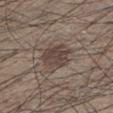Notes:
– biopsy status: imaged on a skin check; not biopsied
– subject: male, aged approximately 35
– site: the left lower leg
– lesion size: ≈4.5 mm
– image-analysis metrics: an area of roughly 12 mm², a shape eccentricity near 0.7, and two-axis asymmetry of about 0.25; a border-irregularity rating of about 2.5/10, internal color variation of about 3 on a 0–10 scale, and a peripheral color-asymmetry measure near 1; a nevus-likeness score of about 90/100 and a detector confidence of about 90 out of 100 that the crop contains a lesion
– imaging modality: ~15 mm tile from a whole-body skin photo
– lighting: white-light illumination